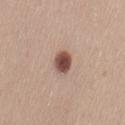| field | value |
|---|---|
| notes | no biopsy performed (imaged during a skin exam) |